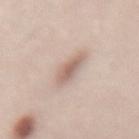Q: Was a biopsy performed?
A: catalogued during a skin exam; not biopsied
Q: Who is the patient?
A: female, aged 63–67
Q: Lesion location?
A: the lower back
Q: What lighting was used for the tile?
A: white-light illumination
Q: What kind of image is this?
A: ~15 mm tile from a whole-body skin photo